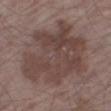notes: imaged on a skin check; not biopsied | location: the leg | size: about 9.5 mm | patient: male, in their mid-60s | image source: total-body-photography crop, ~15 mm field of view.